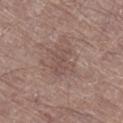notes: catalogued during a skin exam; not biopsied | size: ≈3.5 mm | subject: male, about 70 years old | illumination: white-light | site: the right lower leg | imaging modality: ~15 mm crop, total-body skin-cancer survey.